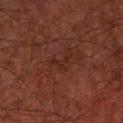From the left forearm. Captured under cross-polarized illumination. A close-up tile cropped from a whole-body skin photograph, about 15 mm across. The lesion's longest dimension is about 2.5 mm. A male subject, aged 63 to 67.